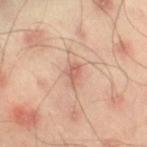biopsy_status: not biopsied; imaged during a skin examination
lighting: cross-polarized
image:
  source: total-body photography crop
  field_of_view_mm: 15
site: right thigh
patient:
  sex: male
  age_approx: 45
lesion_size:
  long_diameter_mm_approx: 2.5
automated_metrics:
  eccentricity: 0.75
  shape_asymmetry: 0.35
  cielab_L: 61
  cielab_a: 22
  cielab_b: 29
  vs_skin_darker_L: 9.0
  vs_skin_contrast_norm: 6.0
  color_variation_0_10: 2.0
  peripheral_color_asymmetry: 0.5